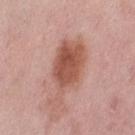Imaged with white-light lighting. A female subject, aged 48 to 52. From the left thigh. A 15 mm close-up tile from a total-body photography series done for melanoma screening. Approximately 7.5 mm at its widest.